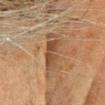The lesion was tiled from a total-body skin photograph and was not biopsied. Measured at roughly 3.5 mm in maximum diameter. Imaged with cross-polarized lighting. A roughly 15 mm field-of-view crop from a total-body skin photograph. The lesion is located on the head or neck. A female patient, aged around 50.